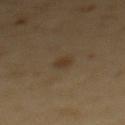Q: Was this lesion biopsied?
A: total-body-photography surveillance lesion; no biopsy
Q: What is the anatomic site?
A: the back
Q: What kind of image is this?
A: total-body-photography crop, ~15 mm field of view
Q: Who is the patient?
A: female, aged 53 to 57
Q: Lesion size?
A: ~2 mm (longest diameter)